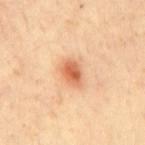Q: Was this lesion biopsied?
A: no biopsy performed (imaged during a skin exam)
Q: Patient demographics?
A: male, aged around 30
Q: Automated lesion metrics?
A: a mean CIELAB color near L≈63 a*≈27 b*≈38 and a normalized lesion–skin contrast near 8.5; a border-irregularity index near 1.5/10
Q: Illumination type?
A: cross-polarized illumination
Q: What kind of image is this?
A: 15 mm crop, total-body photography
Q: What is the anatomic site?
A: the back